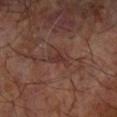Captured during whole-body skin photography for melanoma surveillance; the lesion was not biopsied.
From the right forearm.
A male subject, approximately 65 years of age.
A close-up tile cropped from a whole-body skin photograph, about 15 mm across.